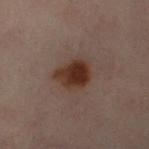Imaged during a routine full-body skin examination; the lesion was not biopsied and no histopathology is available. This image is a 15 mm lesion crop taken from a total-body photograph. The patient is a female aged 58 to 62. Imaged with cross-polarized lighting. The lesion is on the left leg. Automated image analysis of the tile measured a footprint of about 11 mm² and two-axis asymmetry of about 0.25. And it measured about 10 CIELAB-L* units darker than the surrounding skin and a normalized lesion–skin contrast near 11. And it measured a border-irregularity rating of about 2/10 and a within-lesion color-variation index near 4/10.